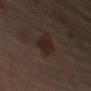Captured during whole-body skin photography for melanoma surveillance; the lesion was not biopsied. A female subject, about 65 years old. A roughly 15 mm field-of-view crop from a total-body skin photograph. Located on the left arm. The total-body-photography lesion software estimated a lesion-to-skin contrast of about 8.5 (normalized; higher = more distinct). The analysis additionally found a border-irregularity rating of about 1.5/10, a color-variation rating of about 2.5/10, and peripheral color asymmetry of about 1. Approximately 3 mm at its widest.